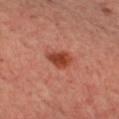Captured during whole-body skin photography for melanoma surveillance; the lesion was not biopsied. Cropped from a total-body skin-imaging series; the visible field is about 15 mm. The lesion is located on the chest. About 3.5 mm across. A female subject, aged around 45.